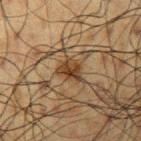Impression: The lesion was photographed on a routine skin check and not biopsied; there is no pathology result.